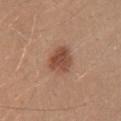Case summary:
• notes · catalogued during a skin exam; not biopsied
• lesion diameter · about 3.5 mm
• lighting · white-light
• subject · male, about 30 years old
• image source · ~15 mm tile from a whole-body skin photo
• anatomic site · the mid back
• automated metrics · an average lesion color of about L≈48 a*≈22 b*≈29 (CIELAB), about 12 CIELAB-L* units darker than the surrounding skin, and a normalized lesion–skin contrast near 8.5; a border-irregularity index near 2/10, a within-lesion color-variation index near 3.5/10, and a peripheral color-asymmetry measure near 1; an automated nevus-likeness rating near 95 out of 100